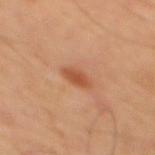<record>
  <biopsy_status>not biopsied; imaged during a skin examination</biopsy_status>
  <lesion_size>
    <long_diameter_mm_approx>3.0</long_diameter_mm_approx>
  </lesion_size>
  <site>back</site>
  <image>
    <source>total-body photography crop</source>
    <field_of_view_mm>15</field_of_view_mm>
  </image>
  <lighting>cross-polarized</lighting>
  <patient>
    <sex>male</sex>
    <age_approx>65</age_approx>
  </patient>
</record>